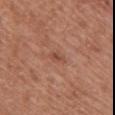<case>
<biopsy_status>not biopsied; imaged during a skin examination</biopsy_status>
<site>right upper arm</site>
<lighting>white-light</lighting>
<image>
  <source>total-body photography crop</source>
  <field_of_view_mm>15</field_of_view_mm>
</image>
<patient>
  <sex>female</sex>
  <age_approx>65</age_approx>
</patient>
</case>